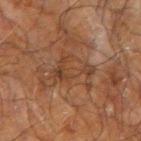Recorded during total-body skin imaging; not selected for excision or biopsy.
On the left forearm.
A 15 mm close-up tile from a total-body photography series done for melanoma screening.
A male patient, aged approximately 70.
The total-body-photography lesion software estimated an average lesion color of about L≈33 a*≈17 b*≈26 (CIELAB), a lesion–skin lightness drop of about 6, and a lesion-to-skin contrast of about 5.5 (normalized; higher = more distinct). The software also gave a peripheral color-asymmetry measure near 0.5. The analysis additionally found a classifier nevus-likeness of about 0/100 and a lesion-detection confidence of about 65/100.
Measured at roughly 4.5 mm in maximum diameter.